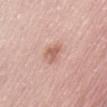Assessment:
Recorded during total-body skin imaging; not selected for excision or biopsy.
Clinical summary:
From the abdomen. A region of skin cropped from a whole-body photographic capture, roughly 15 mm wide. A male subject, aged 53–57. This is a white-light tile. Approximately 3 mm at its widest.